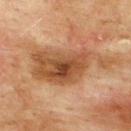Impression: Captured during whole-body skin photography for melanoma surveillance; the lesion was not biopsied. Context: The tile uses cross-polarized illumination. A 15 mm close-up tile from a total-body photography series done for melanoma screening. A male subject, in their mid- to late 70s. The total-body-photography lesion software estimated a lesion area of about 28 mm², an outline eccentricity of about 0.9 (0 = round, 1 = elongated), and a shape-asymmetry score of about 0.4 (0 = symmetric). It also reported an average lesion color of about L≈42 a*≈19 b*≈31 (CIELAB) and about 10 CIELAB-L* units darker than the surrounding skin. And it measured a nevus-likeness score of about 0/100. From the upper back.